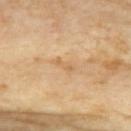{
  "biopsy_status": "not biopsied; imaged during a skin examination",
  "image": {
    "source": "total-body photography crop",
    "field_of_view_mm": 15
  },
  "site": "chest",
  "patient": {
    "sex": "female",
    "age_approx": 75
  }
}